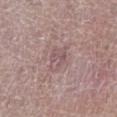Captured during whole-body skin photography for melanoma surveillance; the lesion was not biopsied. A region of skin cropped from a whole-body photographic capture, roughly 15 mm wide. The subject is a female aged 38 to 42. The lesion is located on the left lower leg. Approximately 3.5 mm at its widest. The total-body-photography lesion software estimated a footprint of about 6.5 mm², a shape eccentricity near 0.65, and a symmetry-axis asymmetry near 0.3. The software also gave internal color variation of about 3.5 on a 0–10 scale and a peripheral color-asymmetry measure near 1.5. The software also gave an automated nevus-likeness rating near 0 out of 100 and a lesion-detection confidence of about 85/100. The tile uses white-light illumination.